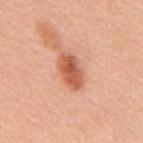No biopsy was performed on this lesion — it was imaged during a full skin examination and was not determined to be concerning.
Automated tile analysis of the lesion measured a shape eccentricity near 0.85. The analysis additionally found a lesion color around L≈59 a*≈29 b*≈35 in CIELAB, roughly 14 lightness units darker than nearby skin, and a lesion-to-skin contrast of about 9 (normalized; higher = more distinct). The software also gave an automated nevus-likeness rating near 95 out of 100.
A region of skin cropped from a whole-body photographic capture, roughly 15 mm wide.
Approximately 4.5 mm at its widest.
A male subject, aged around 65.
Located on the upper back.